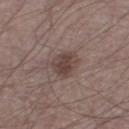Q: Was this lesion biopsied?
A: imaged on a skin check; not biopsied
Q: Illumination type?
A: white-light illumination
Q: What are the patient's age and sex?
A: male, aged around 55
Q: What is the anatomic site?
A: the right lower leg
Q: What kind of image is this?
A: total-body-photography crop, ~15 mm field of view
Q: Lesion size?
A: about 4 mm